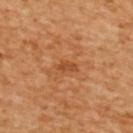Clinical impression:
Imaged during a routine full-body skin examination; the lesion was not biopsied and no histopathology is available.
Acquisition and patient details:
A 15 mm close-up tile from a total-body photography series done for melanoma screening. The total-body-photography lesion software estimated a lesion color around L≈50 a*≈28 b*≈42 in CIELAB and a normalized lesion–skin contrast near 6. It also reported a detector confidence of about 100 out of 100 that the crop contains a lesion. The lesion is on the upper back. The subject is a female in their mid- to late 50s. The lesion's longest dimension is about 2.5 mm. Imaged with cross-polarized lighting.